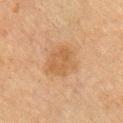Part of a total-body skin-imaging series; this lesion was reviewed on a skin check and was not flagged for biopsy. From the left upper arm. The subject is a male in their mid-60s. Longest diameter approximately 4.5 mm. Cropped from a total-body skin-imaging series; the visible field is about 15 mm.